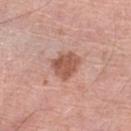Image and clinical context: A male patient aged around 80. From the left lower leg. The tile uses white-light illumination. A 15 mm crop from a total-body photograph taken for skin-cancer surveillance.